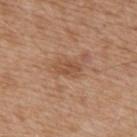<record>
  <biopsy_status>not biopsied; imaged during a skin examination</biopsy_status>
  <lighting>white-light</lighting>
  <site>upper back</site>
  <image>
    <source>total-body photography crop</source>
    <field_of_view_mm>15</field_of_view_mm>
  </image>
  <lesion_size>
    <long_diameter_mm_approx>3.0</long_diameter_mm_approx>
  </lesion_size>
  <patient>
    <sex>male</sex>
    <age_approx>75</age_approx>
  </patient>
</record>